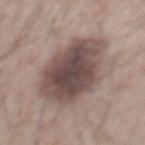Clinical impression: Part of a total-body skin-imaging series; this lesion was reviewed on a skin check and was not flagged for biopsy. Acquisition and patient details: Longest diameter approximately 7.5 mm. The lesion is located on the front of the torso. The lesion-visualizer software estimated a shape eccentricity near 0.5 and a symmetry-axis asymmetry near 0.15. And it measured an average lesion color of about L≈47 a*≈15 b*≈19 (CIELAB), roughly 15 lightness units darker than nearby skin, and a lesion-to-skin contrast of about 10.5 (normalized; higher = more distinct). It also reported a border-irregularity index near 2/10 and a color-variation rating of about 5.5/10. A region of skin cropped from a whole-body photographic capture, roughly 15 mm wide. A male patient in their mid- to late 60s. Captured under white-light illumination.